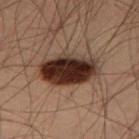Notes:
- workup: total-body-photography surveillance lesion; no biopsy
- anatomic site: the leg
- lesion size: ~7 mm (longest diameter)
- subject: male, approximately 55 years of age
- illumination: cross-polarized
- imaging modality: total-body-photography crop, ~15 mm field of view